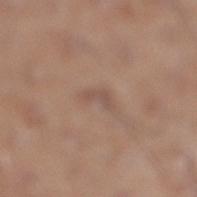Recorded during total-body skin imaging; not selected for excision or biopsy. A 15 mm crop from a total-body photograph taken for skin-cancer surveillance. Imaged with white-light lighting. A male patient approximately 60 years of age. The lesion is on the right lower leg. The recorded lesion diameter is about 2.5 mm.